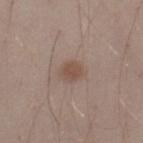Q: Was a biopsy performed?
A: catalogued during a skin exam; not biopsied
Q: What is the imaging modality?
A: 15 mm crop, total-body photography
Q: Lesion location?
A: the left thigh
Q: Patient demographics?
A: male, about 30 years old